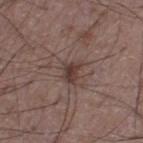Captured during whole-body skin photography for melanoma surveillance; the lesion was not biopsied.
The tile uses white-light illumination.
A male patient, approximately 50 years of age.
On the left thigh.
The lesion's longest dimension is about 3 mm.
Cropped from a total-body skin-imaging series; the visible field is about 15 mm.